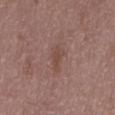No biopsy was performed on this lesion — it was imaged during a full skin examination and was not determined to be concerning. The subject is a male in their 50s. A roughly 15 mm field-of-view crop from a total-body skin photograph. From the mid back.